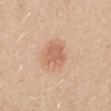Notes:
• image-analysis metrics — a lesion color around L≈63 a*≈22 b*≈34 in CIELAB, about 9 CIELAB-L* units darker than the surrounding skin, and a normalized border contrast of about 6; a nevus-likeness score of about 80/100
• anatomic site — the mid back
• image source — ~15 mm crop, total-body skin-cancer survey
• patient — female, in their 40s
• lesion diameter — ~3.5 mm (longest diameter)